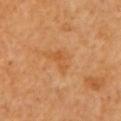Recorded during total-body skin imaging; not selected for excision or biopsy. This is a cross-polarized tile. This image is a 15 mm lesion crop taken from a total-body photograph. The lesion is located on the left upper arm. The lesion-visualizer software estimated a border-irregularity rating of about 4.5/10 and a within-lesion color-variation index near 0.5/10. The analysis additionally found an automated nevus-likeness rating near 0 out of 100 and a lesion-detection confidence of about 100/100. A female subject about 55 years old. The lesion's longest dimension is about 3 mm.